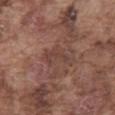<lesion>
  <biopsy_status>not biopsied; imaged during a skin examination</biopsy_status>
  <image>
    <source>total-body photography crop</source>
    <field_of_view_mm>15</field_of_view_mm>
  </image>
  <lesion_size>
    <long_diameter_mm_approx>4.0</long_diameter_mm_approx>
  </lesion_size>
  <site>abdomen</site>
  <automated_metrics>
    <area_mm2_approx>5.5</area_mm2_approx>
    <eccentricity>0.9</eccentricity>
    <shape_asymmetry>0.55</shape_asymmetry>
    <border_irregularity_0_10>6.5</border_irregularity_0_10>
    <color_variation_0_10>1.5</color_variation_0_10>
    <peripheral_color_asymmetry>0.5</peripheral_color_asymmetry>
    <nevus_likeness_0_100>0</nevus_likeness_0_100>
    <lesion_detection_confidence_0_100>95</lesion_detection_confidence_0_100>
  </automated_metrics>
  <patient>
    <sex>male</sex>
    <age_approx>75</age_approx>
  </patient>
  <lighting>white-light</lighting>
</lesion>